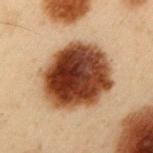biopsy_status: not biopsied; imaged during a skin examination